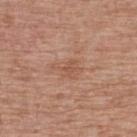Findings:
• subject — male, approximately 80 years of age
• diameter — ≈3.5 mm
• lighting — white-light
• image source — ~15 mm tile from a whole-body skin photo
• image-analysis metrics — a mean CIELAB color near L≈54 a*≈22 b*≈30 and roughly 6 lightness units darker than nearby skin; a border-irregularity index near 4/10, a within-lesion color-variation index near 2/10, and a peripheral color-asymmetry measure near 0.5; an automated nevus-likeness rating near 0 out of 100 and a lesion-detection confidence of about 100/100
• location — the back